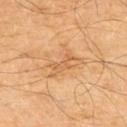location = the chest; automated lesion analysis = a mean CIELAB color near L≈59 a*≈22 b*≈40 and a lesion–skin lightness drop of about 7; diameter = ≈3 mm; subject = male, aged 58–62; illumination = cross-polarized illumination; image = ~15 mm crop, total-body skin-cancer survey.